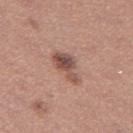Clinical summary: A roughly 15 mm field-of-view crop from a total-body skin photograph. Longest diameter approximately 4.5 mm. Imaged with white-light lighting. A female patient about 30 years old. An algorithmic analysis of the crop reported a border-irregularity rating of about 4.5/10, a within-lesion color-variation index near 6/10, and peripheral color asymmetry of about 2. And it measured a classifier nevus-likeness of about 65/100 and a detector confidence of about 100 out of 100 that the crop contains a lesion. Located on the left thigh.